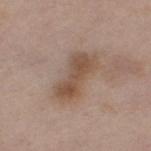The lesion was photographed on a routine skin check and not biopsied; there is no pathology result.
Captured under white-light illumination.
The subject is a female in their mid-60s.
On the left thigh.
Automated image analysis of the tile measured a classifier nevus-likeness of about 0/100 and a lesion-detection confidence of about 100/100.
Cropped from a whole-body photographic skin survey; the tile spans about 15 mm.
Longest diameter approximately 5.5 mm.